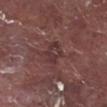<case>
<biopsy_status>not biopsied; imaged during a skin examination</biopsy_status>
<lighting>white-light</lighting>
<image>
  <source>total-body photography crop</source>
  <field_of_view_mm>15</field_of_view_mm>
</image>
<automated_metrics>
  <nevus_likeness_0_100>0</nevus_likeness_0_100>
  <lesion_detection_confidence_0_100>70</lesion_detection_confidence_0_100>
</automated_metrics>
<site>leg</site>
<patient>
  <sex>male</sex>
  <age_approx>75</age_approx>
</patient>
</case>